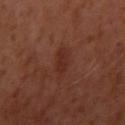notes: imaged on a skin check; not biopsied
body site: the right upper arm
lesion size: ~3.5 mm (longest diameter)
lighting: cross-polarized illumination
image: total-body-photography crop, ~15 mm field of view
patient: female, aged approximately 40